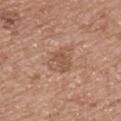Part of a total-body skin-imaging series; this lesion was reviewed on a skin check and was not flagged for biopsy.
Automated tile analysis of the lesion measured a footprint of about 7 mm² and a symmetry-axis asymmetry near 0.3. The analysis additionally found a border-irregularity rating of about 3/10, a within-lesion color-variation index near 3.5/10, and radial color variation of about 1.
A region of skin cropped from a whole-body photographic capture, roughly 15 mm wide.
The patient is a male aged approximately 55.
On the chest.
The recorded lesion diameter is about 3.5 mm.
Imaged with white-light lighting.